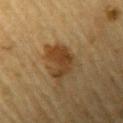Captured during whole-body skin photography for melanoma surveillance; the lesion was not biopsied. A roughly 15 mm field-of-view crop from a total-body skin photograph. The lesion's longest dimension is about 4.5 mm. On the left upper arm. A male subject about 85 years old or older. The tile uses cross-polarized illumination.